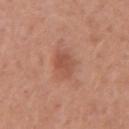workup — no biopsy performed (imaged during a skin exam)
tile lighting — white-light
body site — the left upper arm
automated metrics — an average lesion color of about L≈52 a*≈25 b*≈31 (CIELAB), roughly 8 lightness units darker than nearby skin, and a normalized lesion–skin contrast near 6; a nevus-likeness score of about 45/100 and a lesion-detection confidence of about 100/100
patient — female, roughly 35 years of age
imaging modality — 15 mm crop, total-body photography
diameter — ~3 mm (longest diameter)